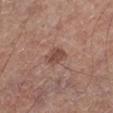  biopsy_status: not biopsied; imaged during a skin examination
  lighting: white-light
  image:
    source: total-body photography crop
    field_of_view_mm: 15
  automated_metrics:
    nevus_likeness_0_100: 65
    lesion_detection_confidence_0_100: 100
  site: leg
  patient:
    sex: male
    age_approx: 70